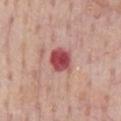lesion size: ~3 mm (longest diameter) | automated lesion analysis: an area of roughly 7 mm² and a shape eccentricity near 0.5; an average lesion color of about L≈49 a*≈34 b*≈23 (CIELAB), a lesion–skin lightness drop of about 17, and a lesion-to-skin contrast of about 11 (normalized; higher = more distinct); a color-variation rating of about 3.5/10 and radial color variation of about 1; lesion-presence confidence of about 100/100 | illumination: white-light | patient: male, roughly 60 years of age | image: ~15 mm tile from a whole-body skin photo | body site: the front of the torso.